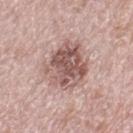Q: What is the imaging modality?
A: total-body-photography crop, ~15 mm field of view
Q: Who is the patient?
A: female, aged 58–62
Q: What is the anatomic site?
A: the left thigh